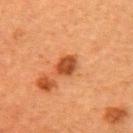{"biopsy_status": "not biopsied; imaged during a skin examination", "lighting": "cross-polarized", "automated_metrics": {"area_mm2_approx": 5.0, "eccentricity": 0.4, "shape_asymmetry": 0.2, "cielab_L": 41, "cielab_a": 27, "cielab_b": 36, "vs_skin_contrast_norm": 9.5, "border_irregularity_0_10": 1.5, "nevus_likeness_0_100": 85, "lesion_detection_confidence_0_100": 100}, "lesion_size": {"long_diameter_mm_approx": 2.5}, "image": {"source": "total-body photography crop", "field_of_view_mm": 15}, "site": "right upper arm", "patient": {"sex": "female", "age_approx": 60}}